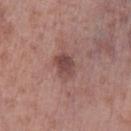Part of a total-body skin-imaging series; this lesion was reviewed on a skin check and was not flagged for biopsy. A male patient, approximately 55 years of age. This is a white-light tile. A 15 mm close-up tile from a total-body photography series done for melanoma screening. Approximately 3 mm at its widest. From the left lower leg.